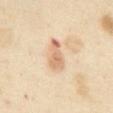follow-up = imaged on a skin check; not biopsied | anatomic site = the abdomen | tile lighting = cross-polarized illumination | patient = female, approximately 55 years of age | automated metrics = a shape eccentricity near 0.85 | diameter = about 3.5 mm | image source = ~15 mm tile from a whole-body skin photo.